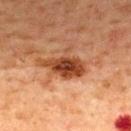Assessment: The lesion was photographed on a routine skin check and not biopsied; there is no pathology result. Context: On the mid back. Longest diameter approximately 5 mm. A close-up tile cropped from a whole-body skin photograph, about 15 mm across. The subject is a male in their mid- to late 60s.